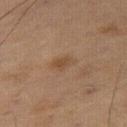The lesion was photographed on a routine skin check and not biopsied; there is no pathology result. The lesion is located on the right thigh. This image is a 15 mm lesion crop taken from a total-body photograph. A male patient aged 53–57. Automated image analysis of the tile measured a lesion color around L≈41 a*≈16 b*≈28 in CIELAB, a lesion–skin lightness drop of about 6, and a lesion-to-skin contrast of about 5.5 (normalized; higher = more distinct). The analysis additionally found a border-irregularity index near 2.5/10 and a within-lesion color-variation index near 2/10. It also reported an automated nevus-likeness rating near 30 out of 100 and a lesion-detection confidence of about 100/100. Imaged with cross-polarized lighting.